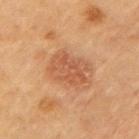Part of a total-body skin-imaging series; this lesion was reviewed on a skin check and was not flagged for biopsy.
The lesion is on the left upper arm.
The patient is a male in their mid- to late 80s or older.
This image is a 15 mm lesion crop taken from a total-body photograph.